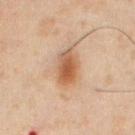Recorded during total-body skin imaging; not selected for excision or biopsy. From the abdomen. A 15 mm close-up tile from a total-body photography series done for melanoma screening. A male subject about 50 years old.